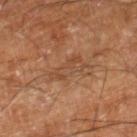Assessment:
No biopsy was performed on this lesion — it was imaged during a full skin examination and was not determined to be concerning.
Image and clinical context:
A male subject aged 58 to 62. Approximately 4.5 mm at its widest. Located on the right lower leg. A 15 mm close-up tile from a total-body photography series done for melanoma screening. Imaged with cross-polarized lighting.